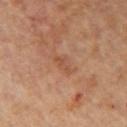Recorded during total-body skin imaging; not selected for excision or biopsy. Imaged with cross-polarized lighting. Cropped from a total-body skin-imaging series; the visible field is about 15 mm. The lesion is located on the right upper arm. A female patient about 45 years old.